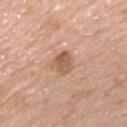Q: Was a biopsy performed?
A: total-body-photography surveillance lesion; no biopsy
Q: What kind of image is this?
A: total-body-photography crop, ~15 mm field of view
Q: What is the anatomic site?
A: the chest
Q: What are the patient's age and sex?
A: male, roughly 45 years of age